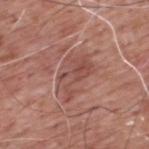This lesion was catalogued during total-body skin photography and was not selected for biopsy.
The patient is a male aged 58 to 62.
About 6 mm across.
Imaged with white-light lighting.
A close-up tile cropped from a whole-body skin photograph, about 15 mm across.
Located on the upper back.